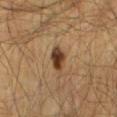Case summary:
* notes · no biopsy performed (imaged during a skin exam)
* site · the mid back
* subject · male, approximately 65 years of age
* acquisition · total-body-photography crop, ~15 mm field of view
* lighting · cross-polarized illumination
* TBP lesion metrics · a lesion color around L≈32 a*≈15 b*≈27 in CIELAB and a lesion-to-skin contrast of about 11.5 (normalized; higher = more distinct)
* size · about 3.5 mm